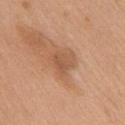Image and clinical context:
This is a white-light tile. A female subject aged 68 to 72. On the chest. The recorded lesion diameter is about 3 mm. A 15 mm crop from a total-body photograph taken for skin-cancer surveillance.